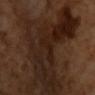Recorded during total-body skin imaging; not selected for excision or biopsy. The recorded lesion diameter is about 3 mm. A male patient in their mid-60s. A 15 mm close-up extracted from a 3D total-body photography capture. This is a cross-polarized tile. Automated tile analysis of the lesion measured an area of roughly 5 mm², an outline eccentricity of about 0.75 (0 = round, 1 = elongated), and a symmetry-axis asymmetry near 0.45. The analysis additionally found a mean CIELAB color near L≈19 a*≈16 b*≈22, a lesion–skin lightness drop of about 3, and a normalized lesion–skin contrast near 5. The software also gave a border-irregularity rating of about 5/10, internal color variation of about 1.5 on a 0–10 scale, and a peripheral color-asymmetry measure near 0.5.